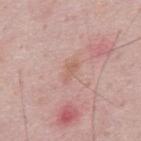The lesion was photographed on a routine skin check and not biopsied; there is no pathology result.
Approximately 2.5 mm at its widest.
On the mid back.
The subject is a male aged 48 to 52.
A close-up tile cropped from a whole-body skin photograph, about 15 mm across.
Captured under white-light illumination.